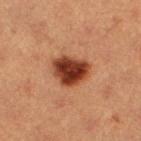This lesion was catalogued during total-body skin photography and was not selected for biopsy.
Approximately 4 mm at its widest.
A 15 mm crop from a total-body photograph taken for skin-cancer surveillance.
The tile uses cross-polarized illumination.
A female patient aged approximately 40.
The lesion is located on the left lower leg.
Automated image analysis of the tile measured an average lesion color of about L≈32 a*≈24 b*≈29 (CIELAB). It also reported a nevus-likeness score of about 100/100.